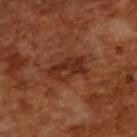Findings:
• biopsy status: no biopsy performed (imaged during a skin exam)
• tile lighting: cross-polarized illumination
• size: ~4.5 mm (longest diameter)
• automated metrics: two-axis asymmetry of about 0.25; a classifier nevus-likeness of about 15/100
• patient: male, roughly 65 years of age
• image source: total-body-photography crop, ~15 mm field of view